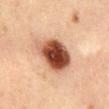Case summary:
- biopsy status: total-body-photography surveillance lesion; no biopsy
- automated metrics: an average lesion color of about L≈51 a*≈25 b*≈33 (CIELAB), roughly 23 lightness units darker than nearby skin, and a lesion-to-skin contrast of about 14 (normalized; higher = more distinct)
- tile lighting: cross-polarized
- subject: male, in their mid- to late 50s
- lesion diameter: about 6 mm
- body site: the back
- acquisition: total-body-photography crop, ~15 mm field of view